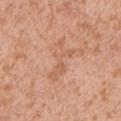Impression:
The lesion was photographed on a routine skin check and not biopsied; there is no pathology result.
Context:
Automated image analysis of the tile measured a shape eccentricity near 0.9 and a symmetry-axis asymmetry near 0.6. The software also gave internal color variation of about 2 on a 0–10 scale and a peripheral color-asymmetry measure near 0.5. The software also gave a nevus-likeness score of about 0/100. A 15 mm close-up tile from a total-body photography series done for melanoma screening. A male subject, approximately 50 years of age. From the left upper arm. Captured under white-light illumination. About 5.5 mm across.